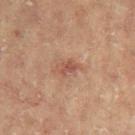Q: Was this lesion biopsied?
A: catalogued during a skin exam; not biopsied
Q: Who is the patient?
A: female, aged 78–82
Q: How was this image acquired?
A: total-body-photography crop, ~15 mm field of view
Q: What is the anatomic site?
A: the left arm
Q: How was the tile lit?
A: cross-polarized illumination